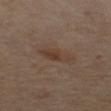<record>
  <patient>
    <sex>female</sex>
    <age_approx>60</age_approx>
  </patient>
  <lighting>cross-polarized</lighting>
  <site>right leg</site>
  <automated_metrics>
    <eccentricity>0.85</eccentricity>
    <shape_asymmetry>0.25</shape_asymmetry>
    <cielab_L>32</cielab_L>
    <cielab_a>14</cielab_a>
    <cielab_b>22</cielab_b>
    <vs_skin_darker_L>6.0</vs_skin_darker_L>
    <color_variation_0_10>1.5</color_variation_0_10>
    <peripheral_color_asymmetry>0.5</peripheral_color_asymmetry>
  </automated_metrics>
  <image>
    <source>total-body photography crop</source>
    <field_of_view_mm>15</field_of_view_mm>
  </image>
</record>